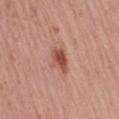Recorded during total-body skin imaging; not selected for excision or biopsy. Located on the right thigh. A roughly 15 mm field-of-view crop from a total-body skin photograph. A female patient, in their mid- to late 50s.